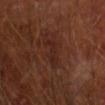Clinical impression: Captured during whole-body skin photography for melanoma surveillance; the lesion was not biopsied. Acquisition and patient details: The recorded lesion diameter is about 3.5 mm. The tile uses cross-polarized illumination. Automated tile analysis of the lesion measured an area of roughly 5.5 mm², a shape eccentricity near 0.9, and two-axis asymmetry of about 0.5. The software also gave a lesion–skin lightness drop of about 4 and a lesion-to-skin contrast of about 5.5 (normalized; higher = more distinct). It also reported a within-lesion color-variation index near 1/10 and a peripheral color-asymmetry measure near 0.5. It also reported an automated nevus-likeness rating near 0 out of 100 and lesion-presence confidence of about 90/100. A close-up tile cropped from a whole-body skin photograph, about 15 mm across. The lesion is located on the right forearm. The patient is a male about 65 years old.